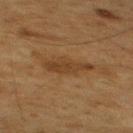Background: The patient is a male about 60 years old. Imaged with cross-polarized lighting. Cropped from a whole-body photographic skin survey; the tile spans about 15 mm. Automated image analysis of the tile measured a lesion color around L≈33 a*≈16 b*≈29 in CIELAB, roughly 6 lightness units darker than nearby skin, and a lesion-to-skin contrast of about 6.5 (normalized; higher = more distinct). The analysis additionally found a nevus-likeness score of about 5/100 and a lesion-detection confidence of about 100/100. The lesion is on the upper back.